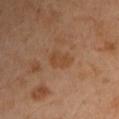Captured during whole-body skin photography for melanoma surveillance; the lesion was not biopsied.